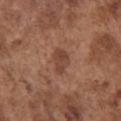Automated tile analysis of the lesion measured an average lesion color of about L≈43 a*≈22 b*≈28 (CIELAB), a lesion–skin lightness drop of about 8, and a normalized border contrast of about 7. It also reported radial color variation of about 1. The analysis additionally found a classifier nevus-likeness of about 40/100 and lesion-presence confidence of about 100/100. A lesion tile, about 15 mm wide, cut from a 3D total-body photograph. About 3 mm across. A male patient, roughly 75 years of age. Captured under white-light illumination. The lesion is located on the chest.